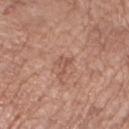biopsy_status: not biopsied; imaged during a skin examination
lighting: white-light
site: right upper arm
image:
  source: total-body photography crop
  field_of_view_mm: 15
patient:
  sex: female
  age_approx: 75
lesion_size:
  long_diameter_mm_approx: 2.5